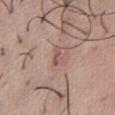  biopsy_status: not biopsied; imaged during a skin examination
  image:
    source: total-body photography crop
    field_of_view_mm: 15
  lighting: white-light
  site: abdomen
  patient:
    sex: female
    age_approx: 45